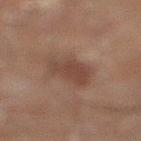Findings:
• workup: catalogued during a skin exam; not biopsied
• lesion diameter: about 5.5 mm
• acquisition: ~15 mm crop, total-body skin-cancer survey
• patient: male, aged 58 to 62
• site: the left leg
• lighting: cross-polarized